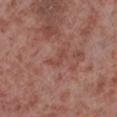Q: Is there a histopathology result?
A: catalogued during a skin exam; not biopsied
Q: What kind of image is this?
A: ~15 mm tile from a whole-body skin photo
Q: Lesion size?
A: ≈3 mm
Q: Patient demographics?
A: male, aged 53–57
Q: What did automated image analysis measure?
A: a footprint of about 3 mm², a shape eccentricity near 0.85, and a shape-asymmetry score of about 0.5 (0 = symmetric); a border-irregularity index near 5.5/10 and a within-lesion color-variation index near 0/10; an automated nevus-likeness rating near 0 out of 100 and lesion-presence confidence of about 100/100
Q: Illumination type?
A: white-light
Q: Where on the body is the lesion?
A: the left lower leg